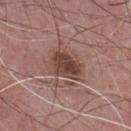Impression: The lesion was tiled from a total-body skin photograph and was not biopsied. Image and clinical context: The patient is a male aged approximately 75. A close-up tile cropped from a whole-body skin photograph, about 15 mm across. Imaged with white-light lighting. Longest diameter approximately 4 mm. Located on the chest.